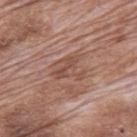  biopsy_status: not biopsied; imaged during a skin examination
  image:
    source: total-body photography crop
    field_of_view_mm: 15
  patient:
    sex: male
    age_approx: 70
  site: mid back